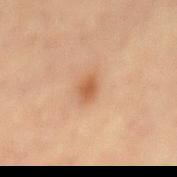No biopsy was performed on this lesion — it was imaged during a full skin examination and was not determined to be concerning.
Located on the back.
A close-up tile cropped from a whole-body skin photograph, about 15 mm across.
A female patient roughly 65 years of age.